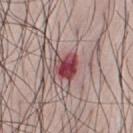  biopsy_status: not biopsied; imaged during a skin examination
  lighting: white-light
  automated_metrics:
    area_mm2_approx: 7.0
    eccentricity: 0.45
    cielab_L: 45
    cielab_a: 30
    cielab_b: 18
    vs_skin_darker_L: 15.0
    vs_skin_contrast_norm: 11.0
  site: chest
  patient:
    sex: male
    age_approx: 35
  image:
    source: total-body photography crop
    field_of_view_mm: 15